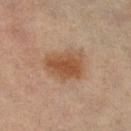Impression:
This lesion was catalogued during total-body skin photography and was not selected for biopsy.
Acquisition and patient details:
The patient is a female aged approximately 50. On the right lower leg. A 15 mm close-up tile from a total-body photography series done for melanoma screening. Captured under cross-polarized illumination.